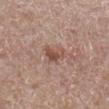The lesion was photographed on a routine skin check and not biopsied; there is no pathology result. On the right lower leg. A male patient, about 65 years old. Automated tile analysis of the lesion measured an area of roughly 4.5 mm². The analysis additionally found lesion-presence confidence of about 100/100. Cropped from a total-body skin-imaging series; the visible field is about 15 mm. The tile uses white-light illumination.